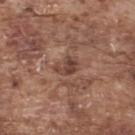biopsy status = no biopsy performed (imaged during a skin exam) | automated lesion analysis = a footprint of about 4 mm², a shape eccentricity near 0.75, and a symmetry-axis asymmetry near 0.45; a classifier nevus-likeness of about 0/100 | imaging modality = total-body-photography crop, ~15 mm field of view | diameter = about 2.5 mm | patient = male, about 75 years old | location = the upper back | lighting = white-light.